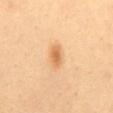follow-up = imaged on a skin check; not biopsied | imaging modality = 15 mm crop, total-body photography | lesion diameter = ~3 mm (longest diameter) | body site = the chest | lighting = cross-polarized illumination | subject = female, about 35 years old.